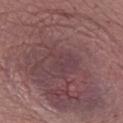workup: total-body-photography surveillance lesion; no biopsy | illumination: white-light illumination | size: ~16.5 mm (longest diameter) | patient: female, aged 48 to 52 | image: 15 mm crop, total-body photography | TBP lesion metrics: an area of roughly 130 mm², a shape eccentricity near 0.7, and two-axis asymmetry of about 0.35; a mean CIELAB color near L≈45 a*≈20 b*≈17, roughly 8 lightness units darker than nearby skin, and a normalized lesion–skin contrast near 6.5; a classifier nevus-likeness of about 0/100 and a detector confidence of about 80 out of 100 that the crop contains a lesion | anatomic site: the chest.